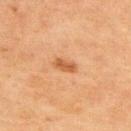| key | value |
|---|---|
| notes | total-body-photography surveillance lesion; no biopsy |
| patient | male, in their 70s |
| image source | total-body-photography crop, ~15 mm field of view |
| tile lighting | cross-polarized |
| anatomic site | the upper back |
| diameter | about 3 mm |